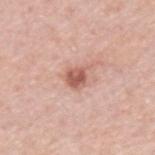* notes — imaged on a skin check; not biopsied
* imaging modality — total-body-photography crop, ~15 mm field of view
* lesion diameter — ≈2.5 mm
* body site — the upper back
* subject — male, roughly 60 years of age
* illumination — white-light
* automated metrics — a shape eccentricity near 0.55 and two-axis asymmetry of about 0.25; a border-irregularity index near 2.5/10 and radial color variation of about 1.5; a nevus-likeness score of about 90/100 and lesion-presence confidence of about 100/100